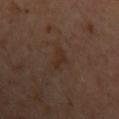Assessment: Captured during whole-body skin photography for melanoma surveillance; the lesion was not biopsied. Image and clinical context: Automated image analysis of the tile measured an average lesion color of about L≈29 a*≈16 b*≈25 (CIELAB), about 5 CIELAB-L* units darker than the surrounding skin, and a lesion-to-skin contrast of about 6 (normalized; higher = more distinct). And it measured a lesion-detection confidence of about 100/100. A lesion tile, about 15 mm wide, cut from a 3D total-body photograph. Located on the left upper arm. This is a cross-polarized tile. A female patient, aged 58 to 62.